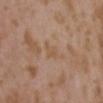The lesion was tiled from a total-body skin photograph and was not biopsied. This is a white-light tile. The lesion's longest dimension is about 3 mm. A 15 mm crop from a total-body photograph taken for skin-cancer surveillance. The lesion is located on the chest. The total-body-photography lesion software estimated a footprint of about 3.5 mm², an eccentricity of roughly 0.85, and two-axis asymmetry of about 0.35. The analysis additionally found a mean CIELAB color near L≈54 a*≈17 b*≈31, a lesion–skin lightness drop of about 5, and a normalized lesion–skin contrast near 5. The analysis additionally found a border-irregularity index near 3.5/10 and a within-lesion color-variation index near 1/10. The software also gave an automated nevus-likeness rating near 0 out of 100. A female patient approximately 35 years of age.